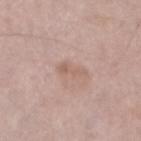Findings:
- follow-up · total-body-photography surveillance lesion; no biopsy
- subject · male, in their mid- to late 60s
- body site · the left lower leg
- lesion diameter · ≈3 mm
- image source · total-body-photography crop, ~15 mm field of view
- lighting · white-light